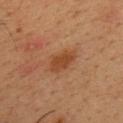Recorded during total-body skin imaging; not selected for excision or biopsy.
About 3.5 mm across.
Captured under cross-polarized illumination.
A male patient, in their mid-30s.
An algorithmic analysis of the crop reported a mean CIELAB color near L≈41 a*≈22 b*≈34, roughly 8 lightness units darker than nearby skin, and a normalized border contrast of about 7.5. The software also gave border irregularity of about 3 on a 0–10 scale, a color-variation rating of about 2/10, and a peripheral color-asymmetry measure near 0.5. And it measured a classifier nevus-likeness of about 70/100 and lesion-presence confidence of about 100/100.
This image is a 15 mm lesion crop taken from a total-body photograph.
Located on the upper back.